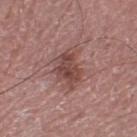This lesion was catalogued during total-body skin photography and was not selected for biopsy. A male patient aged 73 to 77. Captured under white-light illumination. A 15 mm crop from a total-body photograph taken for skin-cancer surveillance. Located on the right lower leg. Automated tile analysis of the lesion measured a border-irregularity index near 5/10, a within-lesion color-variation index near 4/10, and a peripheral color-asymmetry measure near 1. The software also gave an automated nevus-likeness rating near 10 out of 100 and a detector confidence of about 100 out of 100 that the crop contains a lesion.